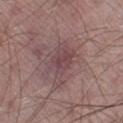biopsy status: catalogued during a skin exam; not biopsied | site: the left thigh | imaging modality: ~15 mm crop, total-body skin-cancer survey | patient: male, approximately 65 years of age.